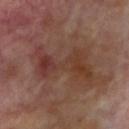This lesion was catalogued during total-body skin photography and was not selected for biopsy. A region of skin cropped from a whole-body photographic capture, roughly 15 mm wide. A female subject in their mid- to late 70s. The lesion's longest dimension is about 8 mm. Located on the left upper arm. This is a cross-polarized tile. Automated image analysis of the tile measured an area of roughly 16 mm² and an eccentricity of roughly 0.95. It also reported a border-irregularity rating of about 9.5/10 and a peripheral color-asymmetry measure near 2.5.